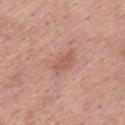Captured during whole-body skin photography for melanoma surveillance; the lesion was not biopsied. Captured under white-light illumination. The patient is a male approximately 55 years of age. On the upper back. Measured at roughly 4 mm in maximum diameter. A 15 mm close-up extracted from a 3D total-body photography capture.